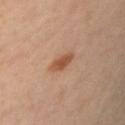The lesion was photographed on a routine skin check and not biopsied; there is no pathology result.
Longest diameter approximately 3 mm.
Captured under cross-polarized illumination.
A male subject aged approximately 55.
The lesion is located on the arm.
The lesion-visualizer software estimated a lesion area of about 4.5 mm². The software also gave about 10 CIELAB-L* units darker than the surrounding skin. The analysis additionally found a border-irregularity rating of about 2.5/10, a within-lesion color-variation index near 2/10, and peripheral color asymmetry of about 0.5.
A 15 mm crop from a total-body photograph taken for skin-cancer surveillance.